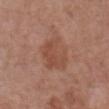Impression: No biopsy was performed on this lesion — it was imaged during a full skin examination and was not determined to be concerning. Image and clinical context: A female patient, aged around 75. Automated image analysis of the tile measured a lesion area of about 13 mm², an outline eccentricity of about 0.6 (0 = round, 1 = elongated), and a symmetry-axis asymmetry near 0.25. And it measured a classifier nevus-likeness of about 0/100. The lesion is on the chest. A roughly 15 mm field-of-view crop from a total-body skin photograph. Measured at roughly 4.5 mm in maximum diameter. Captured under white-light illumination.